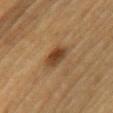Assessment:
The lesion was tiled from a total-body skin photograph and was not biopsied.
Clinical summary:
The subject is a male aged 83 or older. About 3 mm across. Cropped from a whole-body photographic skin survey; the tile spans about 15 mm. Located on the left upper arm. This is a cross-polarized tile.